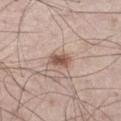Imaged during a routine full-body skin examination; the lesion was not biopsied and no histopathology is available.
The lesion is on the left thigh.
The recorded lesion diameter is about 2.5 mm.
A 15 mm close-up extracted from a 3D total-body photography capture.
A male subject, aged 58 to 62.
Imaged with white-light lighting.